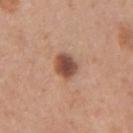This lesion was catalogued during total-body skin photography and was not selected for biopsy.
A lesion tile, about 15 mm wide, cut from a 3D total-body photograph.
This is a white-light tile.
The lesion-visualizer software estimated a nevus-likeness score of about 95/100 and a detector confidence of about 100 out of 100 that the crop contains a lesion.
A female patient approximately 35 years of age.
The lesion is on the left forearm.
Measured at roughly 3 mm in maximum diameter.